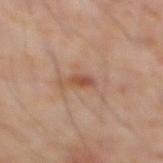biopsy status=imaged on a skin check; not biopsied
anatomic site=the abdomen
tile lighting=cross-polarized
patient=male, in their 60s
image source=15 mm crop, total-body photography
lesion size=≈2.5 mm
TBP lesion metrics=a footprint of about 2.5 mm², a shape eccentricity near 0.9, and two-axis asymmetry of about 0.3; a nevus-likeness score of about 35/100 and a detector confidence of about 100 out of 100 that the crop contains a lesion